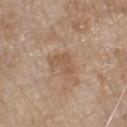biopsy_status: not biopsied; imaged during a skin examination
automated_metrics:
  eccentricity: 0.65
  shape_asymmetry: 0.45
  cielab_L: 55
  cielab_a: 17
  cielab_b: 31
  vs_skin_contrast_norm: 5.5
  border_irregularity_0_10: 4.5
  color_variation_0_10: 2.0
  peripheral_color_asymmetry: 0.5
  nevus_likeness_0_100: 0
lighting: white-light
image:
  source: total-body photography crop
  field_of_view_mm: 15
site: chest
patient:
  sex: male
  age_approx: 80